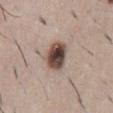| feature | finding |
|---|---|
| body site | the abdomen |
| automated lesion analysis | a footprint of about 9 mm², an outline eccentricity of about 0.6 (0 = round, 1 = elongated), and a shape-asymmetry score of about 0.15 (0 = symmetric); a border-irregularity index near 1.5/10 and a within-lesion color-variation index near 8.5/10 |
| lesion size | ≈3.5 mm |
| subject | male, aged 48–52 |
| image source | ~15 mm tile from a whole-body skin photo |